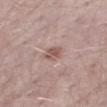This lesion was catalogued during total-body skin photography and was not selected for biopsy.
The subject is a male aged 48 to 52.
The tile uses white-light illumination.
A 15 mm crop from a total-body photograph taken for skin-cancer surveillance.
Located on the right thigh.